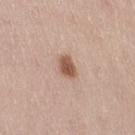  biopsy_status: not biopsied; imaged during a skin examination
  site: right thigh
  patient:
    sex: female
    age_approx: 40
  image:
    source: total-body photography crop
    field_of_view_mm: 15